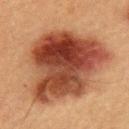This lesion was catalogued during total-body skin photography and was not selected for biopsy.
A male patient, roughly 50 years of age.
A region of skin cropped from a whole-body photographic capture, roughly 15 mm wide.
About 11 mm across.
Automated tile analysis of the lesion measured a footprint of about 65 mm², an eccentricity of roughly 0.6, and a shape-asymmetry score of about 0.25 (0 = symmetric). And it measured a mean CIELAB color near L≈37 a*≈22 b*≈28 and about 15 CIELAB-L* units darker than the surrounding skin. The software also gave a border-irregularity rating of about 3/10, a color-variation rating of about 9/10, and a peripheral color-asymmetry measure near 3. It also reported a classifier nevus-likeness of about 95/100 and a lesion-detection confidence of about 100/100.
From the left upper arm.
The tile uses cross-polarized illumination.